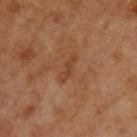Clinical impression:
Imaged during a routine full-body skin examination; the lesion was not biopsied and no histopathology is available.
Clinical summary:
Automated tile analysis of the lesion measured a shape eccentricity near 0.95 and a symmetry-axis asymmetry near 0.3. The analysis additionally found an average lesion color of about L≈31 a*≈17 b*≈26 (CIELAB) and a normalized border contrast of about 5. The analysis additionally found a border-irregularity rating of about 4/10 and a color-variation rating of about 0/10. A region of skin cropped from a whole-body photographic capture, roughly 15 mm wide. A male subject, aged around 60. Captured under cross-polarized illumination. The lesion is located on the upper back.